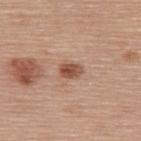Notes:
• workup: total-body-photography surveillance lesion; no biopsy
• patient: female, aged around 60
• location: the upper back
• illumination: white-light
• lesion size: ~2.5 mm (longest diameter)
• acquisition: total-body-photography crop, ~15 mm field of view